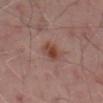No biopsy was performed on this lesion — it was imaged during a full skin examination and was not determined to be concerning.
Automated image analysis of the tile measured an eccentricity of roughly 0.8. And it measured a lesion color around L≈42 a*≈21 b*≈26 in CIELAB and about 9 CIELAB-L* units darker than the surrounding skin. The analysis additionally found a nevus-likeness score of about 80/100 and a detector confidence of about 100 out of 100 that the crop contains a lesion.
Imaged with cross-polarized lighting.
The lesion is located on the front of the torso.
A male subject in their 50s.
A 15 mm crop from a total-body photograph taken for skin-cancer surveillance.
The lesion's longest dimension is about 3 mm.